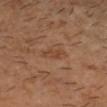Impression: This lesion was catalogued during total-body skin photography and was not selected for biopsy. Background: A roughly 15 mm field-of-view crop from a total-body skin photograph. A male patient, in their mid- to late 40s. From the head or neck.